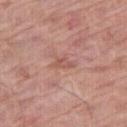biopsy status = no biopsy performed (imaged during a skin exam)
body site = the right thigh
tile lighting = white-light
image source = ~15 mm tile from a whole-body skin photo
automated metrics = a symmetry-axis asymmetry near 0.45; a lesion color around L≈55 a*≈24 b*≈27 in CIELAB, a lesion–skin lightness drop of about 7, and a normalized border contrast of about 5; a border-irregularity rating of about 4.5/10, a within-lesion color-variation index near 1/10, and a peripheral color-asymmetry measure near 0
patient = male, aged 68 to 72